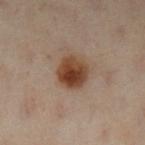<lesion>
<biopsy_status>not biopsied; imaged during a skin examination</biopsy_status>
<lesion_size>
  <long_diameter_mm_approx>3.5</long_diameter_mm_approx>
</lesion_size>
<site>leg</site>
<lighting>cross-polarized</lighting>
<image>
  <source>total-body photography crop</source>
  <field_of_view_mm>15</field_of_view_mm>
</image>
<patient>
  <sex>female</sex>
  <age_approx>60</age_approx>
</patient>
</lesion>